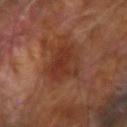Q: What are the patient's age and sex?
A: male, in their mid-60s
Q: Where on the body is the lesion?
A: the right forearm
Q: How large is the lesion?
A: ≈5 mm
Q: What is the imaging modality?
A: 15 mm crop, total-body photography